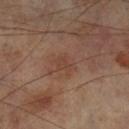Assessment: Imaged during a routine full-body skin examination; the lesion was not biopsied and no histopathology is available. Acquisition and patient details: A lesion tile, about 15 mm wide, cut from a 3D total-body photograph. The tile uses cross-polarized illumination. Longest diameter approximately 3 mm. The lesion is on the left lower leg. A male patient approximately 70 years of age.